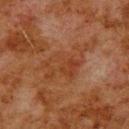{
  "automated_metrics": {
    "color_variation_0_10": 4.5,
    "peripheral_color_asymmetry": 1.5
  },
  "image": {
    "source": "total-body photography crop",
    "field_of_view_mm": 15
  },
  "site": "upper back",
  "lesion_size": {
    "long_diameter_mm_approx": 7.0
  },
  "patient": {
    "sex": "male",
    "age_approx": 80
  }
}